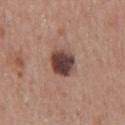follow-up: imaged on a skin check; not biopsied
acquisition: ~15 mm crop, total-body skin-cancer survey
lighting: white-light illumination
location: the chest
patient: male, aged 63–67
diameter: ≈3.5 mm